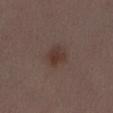{"biopsy_status": "not biopsied; imaged during a skin examination", "lighting": "white-light", "automated_metrics": {"area_mm2_approx": 6.0, "shape_asymmetry": 0.2, "vs_skin_darker_L": 7.0, "vs_skin_contrast_norm": 7.0}, "image": {"source": "total-body photography crop", "field_of_view_mm": 15}, "site": "abdomen", "patient": {"sex": "female", "age_approx": 30}}